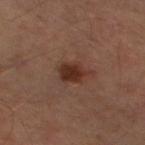{"biopsy_status": "not biopsied; imaged during a skin examination", "patient": {"sex": "male", "age_approx": 55}, "site": "left thigh", "image": {"source": "total-body photography crop", "field_of_view_mm": 15}}